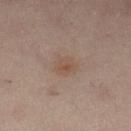Case summary:
• workup: total-body-photography surveillance lesion; no biopsy
• tile lighting: cross-polarized illumination
• location: the left lower leg
• patient: female, approximately 55 years of age
• size: ~2.5 mm (longest diameter)
• automated metrics: a classifier nevus-likeness of about 5/100
• imaging modality: ~15 mm crop, total-body skin-cancer survey